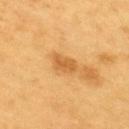Assessment: This lesion was catalogued during total-body skin photography and was not selected for biopsy. Clinical summary: From the upper back. A 15 mm close-up tile from a total-body photography series done for melanoma screening. The subject is a male aged 58 to 62.